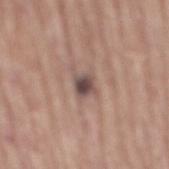<tbp_lesion>
<biopsy_status>not biopsied; imaged during a skin examination</biopsy_status>
<lesion_size>
  <long_diameter_mm_approx>3.5</long_diameter_mm_approx>
</lesion_size>
<patient>
  <sex>male</sex>
  <age_approx>75</age_approx>
</patient>
<image>
  <source>total-body photography crop</source>
  <field_of_view_mm>15</field_of_view_mm>
</image>
<site>mid back</site>
<automated_metrics>
  <cielab_L>49</cielab_L>
  <cielab_a>16</cielab_a>
  <cielab_b>20</cielab_b>
  <vs_skin_contrast_norm>9.0</vs_skin_contrast_norm>
  <border_irregularity_0_10>3.5</border_irregularity_0_10>
  <color_variation_0_10>5.0</color_variation_0_10>
  <peripheral_color_asymmetry>1.5</peripheral_color_asymmetry>
  <lesion_detection_confidence_0_100>100</lesion_detection_confidence_0_100>
</automated_metrics>
</tbp_lesion>